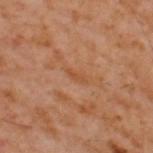Findings:
– biopsy status · no biopsy performed (imaged during a skin exam)
– patient · male, aged 58 to 62
– image-analysis metrics · a lesion area of about 2.5 mm², an eccentricity of roughly 0.9, and two-axis asymmetry of about 0.25; an average lesion color of about L≈47 a*≈22 b*≈35 (CIELAB), roughly 5 lightness units darker than nearby skin, and a normalized lesion–skin contrast near 5; border irregularity of about 3 on a 0–10 scale, a color-variation rating of about 0/10, and peripheral color asymmetry of about 0; an automated nevus-likeness rating near 0 out of 100 and a lesion-detection confidence of about 95/100
– site · the upper back
– illumination · cross-polarized illumination
– image · ~15 mm crop, total-body skin-cancer survey